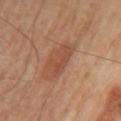Notes:
• biopsy status · total-body-photography surveillance lesion; no biopsy
• illumination · cross-polarized illumination
• patient · male, aged 53–57
• site · the left upper arm
• image source · 15 mm crop, total-body photography
• automated lesion analysis · a footprint of about 7.5 mm², a shape eccentricity near 0.8, and a shape-asymmetry score of about 0.25 (0 = symmetric); a lesion color around L≈49 a*≈24 b*≈32 in CIELAB, a lesion–skin lightness drop of about 7, and a normalized lesion–skin contrast near 5.5; a classifier nevus-likeness of about 100/100 and lesion-presence confidence of about 100/100